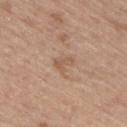Assessment: Part of a total-body skin-imaging series; this lesion was reviewed on a skin check and was not flagged for biopsy. Context: This is a white-light tile. A 15 mm crop from a total-body photograph taken for skin-cancer surveillance. The lesion is on the right thigh. Automated image analysis of the tile measured border irregularity of about 4.5 on a 0–10 scale and a color-variation rating of about 1/10. The software also gave a classifier nevus-likeness of about 0/100. The recorded lesion diameter is about 2.5 mm. A male subject, aged 68–72.